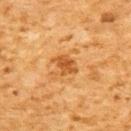  biopsy_status: not biopsied; imaged during a skin examination
  patient:
    sex: male
    age_approx: 60
  site: back
  image:
    source: total-body photography crop
    field_of_view_mm: 15